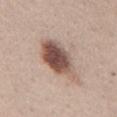Imaged during a routine full-body skin examination; the lesion was not biopsied and no histopathology is available.
Cropped from a total-body skin-imaging series; the visible field is about 15 mm.
The lesion is located on the mid back.
An algorithmic analysis of the crop reported a footprint of about 16 mm², a shape eccentricity near 0.85, and two-axis asymmetry of about 0.2. The analysis additionally found a lesion color around L≈52 a*≈18 b*≈24 in CIELAB, roughly 17 lightness units darker than nearby skin, and a lesion-to-skin contrast of about 11.5 (normalized; higher = more distinct). And it measured a border-irregularity index near 3/10, a color-variation rating of about 7.5/10, and radial color variation of about 2.5. The analysis additionally found an automated nevus-likeness rating near 100 out of 100.
Captured under white-light illumination.
The patient is a male aged 38–42.